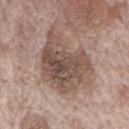follow-up: total-body-photography surveillance lesion; no biopsy
illumination: white-light illumination
image-analysis metrics: a footprint of about 30 mm², an outline eccentricity of about 0.75 (0 = round, 1 = elongated), and a shape-asymmetry score of about 0.35 (0 = symmetric); roughly 12 lightness units darker than nearby skin and a lesion-to-skin contrast of about 8.5 (normalized; higher = more distinct); a border-irregularity index near 4.5/10, a color-variation rating of about 5.5/10, and peripheral color asymmetry of about 1.5; a classifier nevus-likeness of about 0/100 and lesion-presence confidence of about 95/100
subject: male, aged 68 to 72
imaging modality: 15 mm crop, total-body photography
size: about 8 mm
anatomic site: the mid back